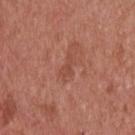Case summary:
* follow-up — total-body-photography surveillance lesion; no biopsy
* image — ~15 mm crop, total-body skin-cancer survey
* patient — male, in their mid- to late 50s
* anatomic site — the back